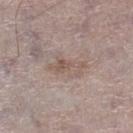Imaged during a routine full-body skin examination; the lesion was not biopsied and no histopathology is available.
A 15 mm crop from a total-body photograph taken for skin-cancer surveillance.
The tile uses white-light illumination.
The subject is a male roughly 65 years of age.
Approximately 4.5 mm at its widest.
The lesion is located on the leg.
The total-body-photography lesion software estimated a lesion area of about 6.5 mm², a shape eccentricity near 0.9, and a symmetry-axis asymmetry near 0.5. It also reported a nevus-likeness score of about 0/100 and a lesion-detection confidence of about 80/100.